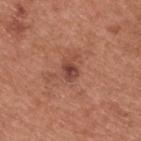Part of a total-body skin-imaging series; this lesion was reviewed on a skin check and was not flagged for biopsy. Imaged with white-light lighting. From the upper back. A male patient approximately 55 years of age. A 15 mm close-up tile from a total-body photography series done for melanoma screening. Automated image analysis of the tile measured an average lesion color of about L≈45 a*≈25 b*≈27 (CIELAB) and a lesion–skin lightness drop of about 10. And it measured a border-irregularity rating of about 3.5/10, internal color variation of about 2.5 on a 0–10 scale, and a peripheral color-asymmetry measure near 1. And it measured a classifier nevus-likeness of about 10/100 and a detector confidence of about 100 out of 100 that the crop contains a lesion.